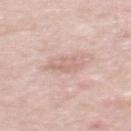Clinical impression: No biopsy was performed on this lesion — it was imaged during a full skin examination and was not determined to be concerning. Clinical summary: The lesion's longest dimension is about 3.5 mm. A male subject aged 53–57. Located on the back. This is a white-light tile. A roughly 15 mm field-of-view crop from a total-body skin photograph.